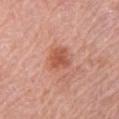Q: What are the patient's age and sex?
A: female, about 60 years old
Q: What is the lesion's diameter?
A: about 2.5 mm
Q: What did automated image analysis measure?
A: a footprint of about 5.5 mm², an outline eccentricity of about 0.3 (0 = round, 1 = elongated), and a symmetry-axis asymmetry near 0.2; an average lesion color of about L≈55 a*≈28 b*≈32 (CIELAB) and roughly 10 lightness units darker than nearby skin; a nevus-likeness score of about 50/100 and a lesion-detection confidence of about 100/100
Q: What is the imaging modality?
A: total-body-photography crop, ~15 mm field of view
Q: What lighting was used for the tile?
A: white-light
Q: Lesion location?
A: the left upper arm